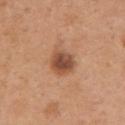{"biopsy_status": "not biopsied; imaged during a skin examination", "site": "arm", "patient": {"sex": "female", "age_approx": 30}, "image": {"source": "total-body photography crop", "field_of_view_mm": 15}}